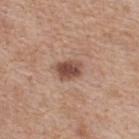The lesion was photographed on a routine skin check and not biopsied; there is no pathology result. This image is a 15 mm lesion crop taken from a total-body photograph. Located on the upper back. Longest diameter approximately 3 mm. A female subject aged 38–42. Captured under white-light illumination. An algorithmic analysis of the crop reported an eccentricity of roughly 0.65. The analysis additionally found an average lesion color of about L≈49 a*≈20 b*≈26 (CIELAB). And it measured lesion-presence confidence of about 100/100.